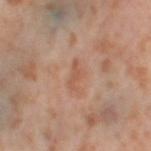<tbp_lesion>
<biopsy_status>not biopsied; imaged during a skin examination</biopsy_status>
<lesion_size>
  <long_diameter_mm_approx>3.0</long_diameter_mm_approx>
</lesion_size>
<automated_metrics>
  <cielab_L>55</cielab_L>
  <cielab_a>22</cielab_a>
  <cielab_b>32</cielab_b>
  <vs_skin_darker_L>7.0</vs_skin_darker_L>
  <vs_skin_contrast_norm>5.5</vs_skin_contrast_norm>
  <nevus_likeness_0_100>0</nevus_likeness_0_100>
  <lesion_detection_confidence_0_100>100</lesion_detection_confidence_0_100>
</automated_metrics>
<patient>
  <sex>female</sex>
  <age_approx>55</age_approx>
</patient>
<image>
  <source>total-body photography crop</source>
  <field_of_view_mm>15</field_of_view_mm>
</image>
<site>leg</site>
</tbp_lesion>